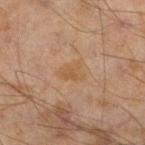| key | value |
|---|---|
| follow-up | total-body-photography surveillance lesion; no biopsy |
| subject | male, roughly 45 years of age |
| tile lighting | cross-polarized |
| image | ~15 mm crop, total-body skin-cancer survey |
| site | the left lower leg |
| lesion size | ≈3 mm |